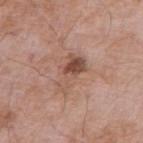Q: Is there a histopathology result?
A: total-body-photography surveillance lesion; no biopsy
Q: What is the anatomic site?
A: the right upper arm
Q: What is the imaging modality?
A: ~15 mm tile from a whole-body skin photo
Q: Lesion size?
A: ~4.5 mm (longest diameter)
Q: What are the patient's age and sex?
A: male, in their mid-70s
Q: How was the tile lit?
A: white-light